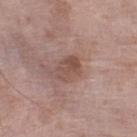Q: Was this lesion biopsied?
A: imaged on a skin check; not biopsied
Q: How was the tile lit?
A: white-light illumination
Q: What kind of image is this?
A: 15 mm crop, total-body photography
Q: How large is the lesion?
A: ≈3 mm
Q: What is the anatomic site?
A: the right lower leg
Q: Patient demographics?
A: female, approximately 75 years of age